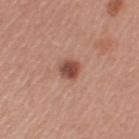Imaged during a routine full-body skin examination; the lesion was not biopsied and no histopathology is available. The recorded lesion diameter is about 2.5 mm. The lesion is on the left upper arm. This is a white-light tile. A female subject aged 48 to 52. A region of skin cropped from a whole-body photographic capture, roughly 15 mm wide.